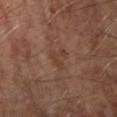This lesion was catalogued during total-body skin photography and was not selected for biopsy. A male patient approximately 65 years of age. The lesion is on the right forearm. The lesion-visualizer software estimated a lesion area of about 3.5 mm², an eccentricity of roughly 0.75, and a symmetry-axis asymmetry near 0.6. It also reported border irregularity of about 7 on a 0–10 scale, a color-variation rating of about 0/10, and peripheral color asymmetry of about 0. It also reported an automated nevus-likeness rating near 0 out of 100 and a detector confidence of about 100 out of 100 that the crop contains a lesion. The lesion's longest dimension is about 3 mm. The tile uses cross-polarized illumination. A roughly 15 mm field-of-view crop from a total-body skin photograph.